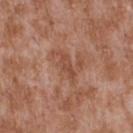Part of a total-body skin-imaging series; this lesion was reviewed on a skin check and was not flagged for biopsy. Located on the upper back. Captured under white-light illumination. A close-up tile cropped from a whole-body skin photograph, about 15 mm across. About 4 mm across. A male subject approximately 45 years of age.